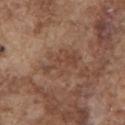The lesion was tiled from a total-body skin photograph and was not biopsied.
A male subject aged 73 to 77.
The lesion is on the chest.
Cropped from a total-body skin-imaging series; the visible field is about 15 mm.
Automated tile analysis of the lesion measured an area of roughly 10 mm², an eccentricity of roughly 0.8, and a symmetry-axis asymmetry near 0.4. It also reported an average lesion color of about L≈44 a*≈19 b*≈28 (CIELAB). It also reported a color-variation rating of about 3/10 and radial color variation of about 1.
The tile uses white-light illumination.
The lesion's longest dimension is about 5 mm.